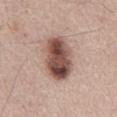biopsy status = imaged on a skin check; not biopsied | imaging modality = 15 mm crop, total-body photography | anatomic site = the abdomen | diameter = ~6.5 mm (longest diameter) | patient = male, roughly 65 years of age | tile lighting = white-light illumination.